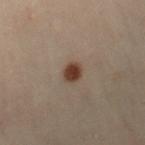<lesion>
<biopsy_status>not biopsied; imaged during a skin examination</biopsy_status>
<lesion_size>
  <long_diameter_mm_approx>2.5</long_diameter_mm_approx>
</lesion_size>
<image>
  <source>total-body photography crop</source>
  <field_of_view_mm>15</field_of_view_mm>
</image>
<site>right thigh</site>
<lighting>cross-polarized</lighting>
<automated_metrics>
  <area_mm2_approx>4.0</area_mm2_approx>
  <eccentricity>0.6</eccentricity>
  <shape_asymmetry>0.15</shape_asymmetry>
  <cielab_L>33</cielab_L>
  <cielab_a>15</cielab_a>
  <cielab_b>22</cielab_b>
  <vs_skin_contrast_norm>11.0</vs_skin_contrast_norm>
  <nevus_likeness_0_100>100</nevus_likeness_0_100>
</automated_metrics>
<patient>
  <sex>female</sex>
  <age_approx>50</age_approx>
</patient>
</lesion>